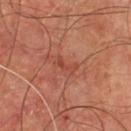biopsy_status: not biopsied; imaged during a skin examination
lesion_size:
  long_diameter_mm_approx: 3.0
image:
  source: total-body photography crop
  field_of_view_mm: 15
automated_metrics:
  cielab_L: 45
  cielab_a: 29
  cielab_b: 31
  vs_skin_darker_L: 7.0
  vs_skin_contrast_norm: 5.5
  nevus_likeness_0_100: 0
patient:
  sex: male
  age_approx: 65
site: chest